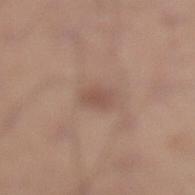biopsy_status: not biopsied; imaged during a skin examination
lighting: white-light
image:
  source: total-body photography crop
  field_of_view_mm: 15
automated_metrics:
  area_mm2_approx: 4.0
  eccentricity: 0.75
  shape_asymmetry: 0.25
  cielab_L: 51
  cielab_a: 18
  cielab_b: 25
  vs_skin_darker_L: 7.0
  vs_skin_contrast_norm: 5.5
  border_irregularity_0_10: 2.0
  peripheral_color_asymmetry: 0.5
  nevus_likeness_0_100: 5
  lesion_detection_confidence_0_100: 100
patient:
  sex: male
  age_approx: 30
site: left lower leg